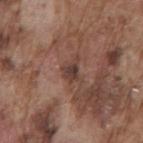Q: Is there a histopathology result?
A: catalogued during a skin exam; not biopsied
Q: What is the imaging modality?
A: total-body-photography crop, ~15 mm field of view
Q: Lesion location?
A: the mid back
Q: What lighting was used for the tile?
A: white-light illumination
Q: Patient demographics?
A: male, aged 73 to 77
Q: Lesion size?
A: ~3 mm (longest diameter)